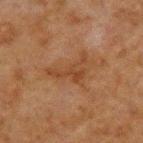No biopsy was performed on this lesion — it was imaged during a full skin examination and was not determined to be concerning.